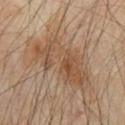notes = total-body-photography surveillance lesion; no biopsy
subject = male, approximately 70 years of age
body site = the chest
acquisition = ~15 mm tile from a whole-body skin photo
lesion diameter = ~8 mm (longest diameter)